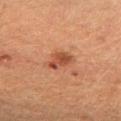Located on the right forearm. A female subject, about 40 years old. The total-body-photography lesion software estimated an area of roughly 4.5 mm² and an outline eccentricity of about 0.75 (0 = round, 1 = elongated). The analysis additionally found a lesion color around L≈40 a*≈25 b*≈30 in CIELAB, a lesion–skin lightness drop of about 10, and a normalized border contrast of about 8. Cropped from a total-body skin-imaging series; the visible field is about 15 mm. This is a cross-polarized tile.